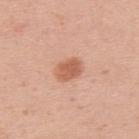Assessment: Captured during whole-body skin photography for melanoma surveillance; the lesion was not biopsied. Image and clinical context: An algorithmic analysis of the crop reported a within-lesion color-variation index near 2.5/10 and peripheral color asymmetry of about 1. The lesion is on the upper back. A roughly 15 mm field-of-view crop from a total-body skin photograph. The lesion's longest dimension is about 3 mm. A female subject about 30 years old. This is a white-light tile.